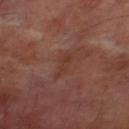– notes — imaged on a skin check; not biopsied
– lesion diameter — ≈3.5 mm
– TBP lesion metrics — a lesion area of about 3.5 mm² and two-axis asymmetry of about 0.4; an average lesion color of about L≈35 a*≈21 b*≈26 (CIELAB), roughly 5 lightness units darker than nearby skin, and a normalized lesion–skin contrast near 5.5; a border-irregularity rating of about 5.5/10, a within-lesion color-variation index near 0.5/10, and a peripheral color-asymmetry measure near 0
– image source — total-body-photography crop, ~15 mm field of view
– body site — the leg
– patient — male, in their 70s